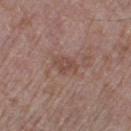Q: Was a biopsy performed?
A: total-body-photography surveillance lesion; no biopsy
Q: Patient demographics?
A: male, roughly 70 years of age
Q: What is the imaging modality?
A: ~15 mm tile from a whole-body skin photo
Q: Lesion location?
A: the right thigh